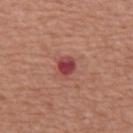Q: Patient demographics?
A: male, in their mid-60s
Q: What is the lesion's diameter?
A: ≈2.5 mm
Q: Lesion location?
A: the abdomen
Q: Illumination type?
A: white-light illumination
Q: What kind of image is this?
A: 15 mm crop, total-body photography
Q: What did automated image analysis measure?
A: a footprint of about 4.5 mm², an eccentricity of roughly 0.5, and two-axis asymmetry of about 0.2; a within-lesion color-variation index near 3.5/10 and peripheral color asymmetry of about 1; a classifier nevus-likeness of about 0/100 and lesion-presence confidence of about 100/100